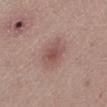Recorded during total-body skin imaging; not selected for excision or biopsy. From the left lower leg. A female patient aged around 20. The lesion's longest dimension is about 3.5 mm. This image is a 15 mm lesion crop taken from a total-body photograph.